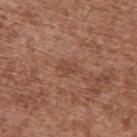Q: Is there a histopathology result?
A: imaged on a skin check; not biopsied
Q: What is the anatomic site?
A: the upper back
Q: What lighting was used for the tile?
A: white-light
Q: What is the imaging modality?
A: ~15 mm tile from a whole-body skin photo
Q: Patient demographics?
A: male, roughly 75 years of age